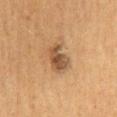biopsy status=catalogued during a skin exam; not biopsied
lesion diameter=~4 mm (longest diameter)
patient=male, approximately 60 years of age
image=15 mm crop, total-body photography
site=the mid back